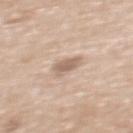The lesion was photographed on a routine skin check and not biopsied; there is no pathology result. The tile uses white-light illumination. From the upper back. A lesion tile, about 15 mm wide, cut from a 3D total-body photograph. The subject is a female in their mid- to late 60s.